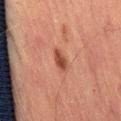The lesion was tiled from a total-body skin photograph and was not biopsied. A male patient, in their mid-60s. On the left thigh. A 15 mm close-up tile from a total-body photography series done for melanoma screening. The recorded lesion diameter is about 3 mm. An algorithmic analysis of the crop reported an outline eccentricity of about 0.85 (0 = round, 1 = elongated). The analysis additionally found a detector confidence of about 100 out of 100 that the crop contains a lesion.